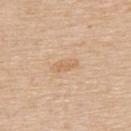follow-up — imaged on a skin check; not biopsied | anatomic site — the upper back | image — total-body-photography crop, ~15 mm field of view | tile lighting — white-light | subject — male, in their mid-60s.